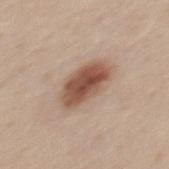  biopsy_status: not biopsied; imaged during a skin examination
  automated_metrics:
    cielab_L: 51
    cielab_a: 20
    cielab_b: 28
    vs_skin_darker_L: 15.0
    vs_skin_contrast_norm: 10.5
    nevus_likeness_0_100: 95
  lighting: white-light
  image:
    source: total-body photography crop
    field_of_view_mm: 15
  patient:
    sex: male
    age_approx: 45
  lesion_size:
    long_diameter_mm_approx: 5.0
  site: mid back